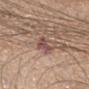follow-up = imaged on a skin check; not biopsied
subject = male, aged 63 to 67
anatomic site = the chest
acquisition = ~15 mm tile from a whole-body skin photo